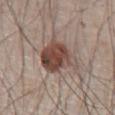Notes:
- workup: no biopsy performed (imaged during a skin exam)
- subject: male, aged 63–67
- lighting: white-light illumination
- image source: 15 mm crop, total-body photography
- anatomic site: the chest
- size: ≈5 mm